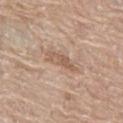Context: About 4 mm across. Captured under white-light illumination. On the left thigh. A lesion tile, about 15 mm wide, cut from a 3D total-body photograph. A female subject approximately 60 years of age.